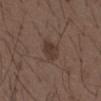This lesion was catalogued during total-body skin photography and was not selected for biopsy.
A male patient, about 50 years old.
About 3 mm across.
This is a white-light tile.
The lesion is located on the chest.
A 15 mm crop from a total-body photograph taken for skin-cancer surveillance.
The total-body-photography lesion software estimated an area of roughly 5.5 mm², a shape eccentricity near 0.7, and a shape-asymmetry score of about 0.25 (0 = symmetric). And it measured a lesion color around L≈36 a*≈15 b*≈22 in CIELAB and about 8 CIELAB-L* units darker than the surrounding skin. It also reported a border-irregularity rating of about 2/10, internal color variation of about 2 on a 0–10 scale, and a peripheral color-asymmetry measure near 1. The software also gave an automated nevus-likeness rating near 55 out of 100 and a lesion-detection confidence of about 100/100.